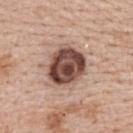Case summary:
– illumination: white-light illumination
– location: the upper back
– lesion size: ~5 mm (longest diameter)
– subject: female, aged 48–52
– image: total-body-photography crop, ~15 mm field of view